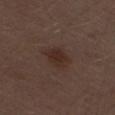biopsy status: imaged on a skin check; not biopsied
automated metrics: a footprint of about 6 mm² and a shape eccentricity near 0.7; a lesion color around L≈27 a*≈16 b*≈21 in CIELAB and roughly 7 lightness units darker than nearby skin; a border-irregularity rating of about 2.5/10 and a within-lesion color-variation index near 2.5/10
tile lighting: white-light
acquisition: 15 mm crop, total-body photography
anatomic site: the right thigh
diameter: about 3 mm
subject: male, about 70 years old